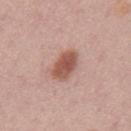Impression: Recorded during total-body skin imaging; not selected for excision or biopsy. Context: The patient is a male aged 43–47. Located on the mid back. The recorded lesion diameter is about 4.5 mm. A 15 mm crop from a total-body photograph taken for skin-cancer surveillance. The tile uses white-light illumination.